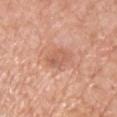Imaged during a routine full-body skin examination; the lesion was not biopsied and no histopathology is available. A male patient, in their 60s. From the chest. A 15 mm crop from a total-body photograph taken for skin-cancer surveillance.